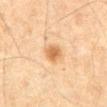biopsy_status: not biopsied; imaged during a skin examination
site: abdomen
patient:
  sex: male
  age_approx: 65
lesion_size:
  long_diameter_mm_approx: 2.5
lighting: cross-polarized
image:
  source: total-body photography crop
  field_of_view_mm: 15
automated_metrics:
  area_mm2_approx: 4.5
  eccentricity: 0.6
  shape_asymmetry: 0.2
  cielab_L: 57
  cielab_a: 19
  cielab_b: 37
  vs_skin_darker_L: 11.0
  vs_skin_contrast_norm: 7.5
  border_irregularity_0_10: 2.0
  peripheral_color_asymmetry: 0.5
  nevus_likeness_0_100: 95
  lesion_detection_confidence_0_100: 100